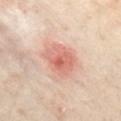Q: Was a biopsy performed?
A: total-body-photography surveillance lesion; no biopsy
Q: What did automated image analysis measure?
A: a lesion area of about 12 mm², an outline eccentricity of about 0.65 (0 = round, 1 = elongated), and two-axis asymmetry of about 0.2; border irregularity of about 2 on a 0–10 scale and radial color variation of about 1.5; a classifier nevus-likeness of about 60/100 and a lesion-detection confidence of about 100/100
Q: What kind of image is this?
A: total-body-photography crop, ~15 mm field of view
Q: What lighting was used for the tile?
A: cross-polarized
Q: Lesion location?
A: the chest
Q: What are the patient's age and sex?
A: female, aged 58–62